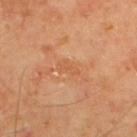Q: Is there a histopathology result?
A: catalogued during a skin exam; not biopsied
Q: What kind of image is this?
A: ~15 mm tile from a whole-body skin photo
Q: What lighting was used for the tile?
A: cross-polarized illumination
Q: What are the patient's age and sex?
A: male, approximately 65 years of age
Q: Where on the body is the lesion?
A: the upper back
Q: Lesion size?
A: about 2.5 mm
Q: Automated lesion metrics?
A: a footprint of about 2.5 mm², an eccentricity of roughly 0.9, and two-axis asymmetry of about 0.35; an average lesion color of about L≈56 a*≈25 b*≈39 (CIELAB), a lesion–skin lightness drop of about 5, and a normalized lesion–skin contrast near 4.5; a within-lesion color-variation index near 0/10 and peripheral color asymmetry of about 0